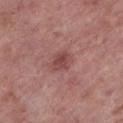Q: Was a biopsy performed?
A: catalogued during a skin exam; not biopsied
Q: How was this image acquired?
A: total-body-photography crop, ~15 mm field of view
Q: What are the patient's age and sex?
A: female, aged approximately 85 or older
Q: What is the anatomic site?
A: the leg
Q: Automated lesion metrics?
A: a lesion area of about 5 mm², a shape eccentricity near 0.65, and two-axis asymmetry of about 0.2